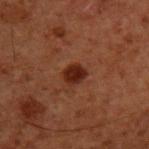No biopsy was performed on this lesion — it was imaged during a full skin examination and was not determined to be concerning.
Cropped from a total-body skin-imaging series; the visible field is about 15 mm.
The lesion is on the upper back.
A male patient, in their 60s.